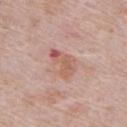lesion_size:
  long_diameter_mm_approx: 4.0
site: abdomen
patient:
  sex: male
  age_approx: 70
automated_metrics:
  vs_skin_contrast_norm: 6.5
  border_irregularity_0_10: 4.0
  peripheral_color_asymmetry: 1.5
  nevus_likeness_0_100: 0
  lesion_detection_confidence_0_100: 100
lighting: white-light
image:
  source: total-body photography crop
  field_of_view_mm: 15